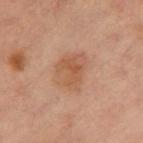Q: Is there a histopathology result?
A: no biopsy performed (imaged during a skin exam)
Q: What kind of image is this?
A: total-body-photography crop, ~15 mm field of view
Q: Lesion size?
A: ~4.5 mm (longest diameter)
Q: What is the anatomic site?
A: the front of the torso
Q: What lighting was used for the tile?
A: cross-polarized
Q: What are the patient's age and sex?
A: female, in their mid- to late 50s